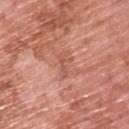Q: Was a biopsy performed?
A: total-body-photography surveillance lesion; no biopsy
Q: Patient demographics?
A: male, roughly 70 years of age
Q: How was this image acquired?
A: ~15 mm crop, total-body skin-cancer survey
Q: Where on the body is the lesion?
A: the upper back
Q: How large is the lesion?
A: about 3 mm
Q: What did automated image analysis measure?
A: an average lesion color of about L≈55 a*≈28 b*≈30 (CIELAB), about 7 CIELAB-L* units darker than the surrounding skin, and a normalized lesion–skin contrast near 5; an automated nevus-likeness rating near 0 out of 100 and a detector confidence of about 100 out of 100 that the crop contains a lesion
Q: Illumination type?
A: white-light illumination